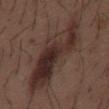Q: Was this lesion biopsied?
A: no biopsy performed (imaged during a skin exam)
Q: What is the anatomic site?
A: the mid back
Q: What kind of image is this?
A: ~15 mm tile from a whole-body skin photo
Q: Patient demographics?
A: male, aged 48–52